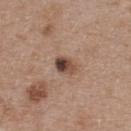Captured during whole-body skin photography for melanoma surveillance; the lesion was not biopsied. The lesion is on the back. The lesion's longest dimension is about 3 mm. This is a white-light tile. A lesion tile, about 15 mm wide, cut from a 3D total-body photograph. The patient is a female roughly 40 years of age.